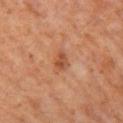Notes:
* notes: no biopsy performed (imaged during a skin exam)
* image source: ~15 mm crop, total-body skin-cancer survey
* patient: male, aged approximately 60
* illumination: cross-polarized
* diameter: ≈2.5 mm
* location: the left thigh
* automated lesion analysis: an area of roughly 4 mm², a shape eccentricity near 0.75, and a shape-asymmetry score of about 0.25 (0 = symmetric); a color-variation rating of about 2.5/10 and a peripheral color-asymmetry measure near 1; lesion-presence confidence of about 100/100